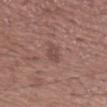Q: Is there a histopathology result?
A: imaged on a skin check; not biopsied
Q: What is the imaging modality?
A: 15 mm crop, total-body photography
Q: How large is the lesion?
A: about 2.5 mm
Q: Where on the body is the lesion?
A: the left forearm
Q: What are the patient's age and sex?
A: male, aged 48 to 52